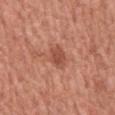This lesion was catalogued during total-body skin photography and was not selected for biopsy.
A male patient in their mid-40s.
A close-up tile cropped from a whole-body skin photograph, about 15 mm across.
Located on the front of the torso.
The lesion-visualizer software estimated roughly 10 lightness units darker than nearby skin and a lesion-to-skin contrast of about 7 (normalized; higher = more distinct). The software also gave a border-irregularity rating of about 1/10, a color-variation rating of about 1.5/10, and radial color variation of about 0.5.